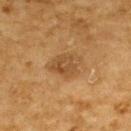Impression:
Recorded during total-body skin imaging; not selected for excision or biopsy.
Clinical summary:
Automated tile analysis of the lesion measured a lesion area of about 9 mm², an eccentricity of roughly 0.7, and a shape-asymmetry score of about 0.25 (0 = symmetric). The software also gave an average lesion color of about L≈43 a*≈17 b*≈34 (CIELAB), about 8 CIELAB-L* units darker than the surrounding skin, and a normalized border contrast of about 6.5. It also reported a classifier nevus-likeness of about 50/100. This is a cross-polarized tile. The lesion is located on the upper back. A male subject aged 83 or older. A 15 mm close-up extracted from a 3D total-body photography capture.